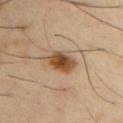| feature | finding |
|---|---|
| biopsy status | total-body-photography surveillance lesion; no biopsy |
| patient | male, in their 40s |
| body site | the chest |
| acquisition | ~15 mm tile from a whole-body skin photo |
| image-analysis metrics | a shape-asymmetry score of about 0.2 (0 = symmetric); a classifier nevus-likeness of about 100/100 and a lesion-detection confidence of about 100/100 |
| diameter | about 3.5 mm |